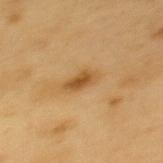• location: the upper back
• acquisition: total-body-photography crop, ~15 mm field of view
• subject: male, about 60 years old